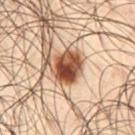notes = imaged on a skin check; not biopsied
size = ~4 mm (longest diameter)
patient = male, about 50 years old
acquisition = 15 mm crop, total-body photography
site = the left thigh